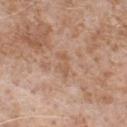Impression: No biopsy was performed on this lesion — it was imaged during a full skin examination and was not determined to be concerning. Background: The lesion is on the chest. Approximately 3.5 mm at its widest. Captured under white-light illumination. A male patient approximately 65 years of age. Cropped from a whole-body photographic skin survey; the tile spans about 15 mm.